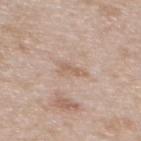Assessment: The lesion was photographed on a routine skin check and not biopsied; there is no pathology result. Background: This is a white-light tile. The lesion's longest dimension is about 3 mm. Automated image analysis of the tile measured an area of roughly 4 mm², a shape eccentricity near 0.85, and two-axis asymmetry of about 0.45. It also reported internal color variation of about 1.5 on a 0–10 scale and a peripheral color-asymmetry measure near 0.5. A female subject aged 28 to 32. A 15 mm crop from a total-body photograph taken for skin-cancer surveillance. From the upper back.